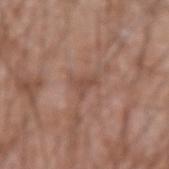Assessment:
No biopsy was performed on this lesion — it was imaged during a full skin examination and was not determined to be concerning.
Clinical summary:
The lesion is located on the left forearm. A 15 mm close-up tile from a total-body photography series done for melanoma screening. The tile uses white-light illumination. A male subject, aged around 60.